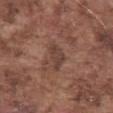Recorded during total-body skin imaging; not selected for excision or biopsy. Located on the abdomen. A lesion tile, about 15 mm wide, cut from a 3D total-body photograph. The subject is a male aged around 75.